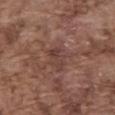Recorded during total-body skin imaging; not selected for excision or biopsy.
Located on the abdomen.
A 15 mm close-up extracted from a 3D total-body photography capture.
About 3 mm across.
The tile uses white-light illumination.
The total-body-photography lesion software estimated an average lesion color of about L≈40 a*≈19 b*≈22 (CIELAB), a lesion–skin lightness drop of about 7, and a normalized border contrast of about 6. And it measured a within-lesion color-variation index near 3.5/10 and radial color variation of about 1.5.
The patient is a male about 75 years old.